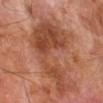Impression: Recorded during total-body skin imaging; not selected for excision or biopsy. Context: On the right forearm. A 15 mm crop from a total-body photograph taken for skin-cancer surveillance. The total-body-photography lesion software estimated a footprint of about 32 mm², a shape eccentricity near 0.7, and a symmetry-axis asymmetry near 0.65. The software also gave a within-lesion color-variation index near 4.5/10 and radial color variation of about 1. A male subject, in their 70s.